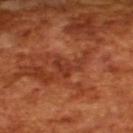Impression:
The lesion was tiled from a total-body skin photograph and was not biopsied.
Background:
A male subject, aged 63 to 67. Measured at roughly 3.5 mm in maximum diameter. A 15 mm close-up tile from a total-body photography series done for melanoma screening.